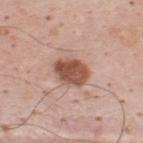Part of a total-body skin-imaging series; this lesion was reviewed on a skin check and was not flagged for biopsy. A male patient aged 33–37. A 15 mm close-up tile from a total-body photography series done for melanoma screening. Measured at roughly 4 mm in maximum diameter. Automated tile analysis of the lesion measured a footprint of about 9.5 mm² and two-axis asymmetry of about 0.15. This is a white-light tile. Located on the upper back.